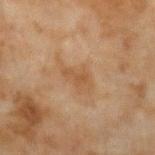This lesion was catalogued during total-body skin photography and was not selected for biopsy. Automated image analysis of the tile measured an area of roughly 2.5 mm², a shape eccentricity near 0.9, and a shape-asymmetry score of about 0.6 (0 = symmetric). And it measured about 6 CIELAB-L* units darker than the surrounding skin. It also reported an automated nevus-likeness rating near 0 out of 100. A male patient, aged 43–47. Captured under cross-polarized illumination. The lesion is on the left forearm. A 15 mm close-up tile from a total-body photography series done for melanoma screening. Measured at roughly 2.5 mm in maximum diameter.